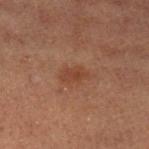notes — total-body-photography surveillance lesion; no biopsy
image-analysis metrics — about 6 CIELAB-L* units darker than the surrounding skin and a lesion-to-skin contrast of about 6 (normalized; higher = more distinct); border irregularity of about 3 on a 0–10 scale, a within-lesion color-variation index near 1.5/10, and peripheral color asymmetry of about 0.5; a classifier nevus-likeness of about 15/100
patient — male, aged 58 to 62
lesion size — about 3 mm
location — the left lower leg
acquisition — 15 mm crop, total-body photography
tile lighting — cross-polarized illumination